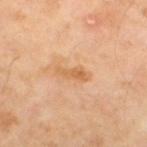Q: Was this lesion biopsied?
A: imaged on a skin check; not biopsied
Q: How large is the lesion?
A: ~3.5 mm (longest diameter)
Q: How was this image acquired?
A: ~15 mm tile from a whole-body skin photo
Q: What is the anatomic site?
A: the right thigh
Q: How was the tile lit?
A: cross-polarized
Q: Who is the patient?
A: male, aged around 55